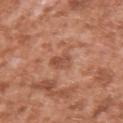{"biopsy_status": "not biopsied; imaged during a skin examination", "patient": {"sex": "male", "age_approx": 45}, "lighting": "white-light", "site": "right upper arm", "automated_metrics": {"area_mm2_approx": 4.0, "eccentricity": 0.75, "shape_asymmetry": 0.25, "cielab_L": 51, "cielab_a": 25, "cielab_b": 32, "vs_skin_darker_L": 8.0, "vs_skin_contrast_norm": 6.0}, "image": {"source": "total-body photography crop", "field_of_view_mm": 15}, "lesion_size": {"long_diameter_mm_approx": 2.5}}